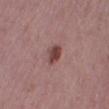A female patient, in their 30s.
This is a white-light tile.
Cropped from a whole-body photographic skin survey; the tile spans about 15 mm.
On the right thigh.